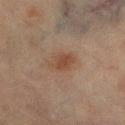| feature | finding |
|---|---|
| workup | no biopsy performed (imaged during a skin exam) |
| subject | female, approximately 55 years of age |
| diameter | about 3.5 mm |
| acquisition | ~15 mm tile from a whole-body skin photo |
| location | the left thigh |